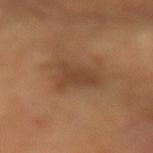Imaged during a routine full-body skin examination; the lesion was not biopsied and no histopathology is available. The tile uses cross-polarized illumination. A female subject, about 55 years old. About 4 mm across. Automated tile analysis of the lesion measured a lesion area of about 9 mm² and two-axis asymmetry of about 0.45. It also reported a mean CIELAB color near L≈44 a*≈20 b*≈32, about 8 CIELAB-L* units darker than the surrounding skin, and a normalized border contrast of about 6.5. The software also gave border irregularity of about 4.5 on a 0–10 scale, a within-lesion color-variation index near 2/10, and radial color variation of about 1. On the right forearm. A 15 mm crop from a total-body photograph taken for skin-cancer surveillance.